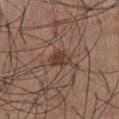Impression: This lesion was catalogued during total-body skin photography and was not selected for biopsy. Acquisition and patient details: The subject is a male approximately 55 years of age. On the abdomen. A region of skin cropped from a whole-body photographic capture, roughly 15 mm wide. Automated tile analysis of the lesion measured a footprint of about 4.5 mm², a shape eccentricity near 0.65, and a shape-asymmetry score of about 0.25 (0 = symmetric). And it measured a lesion-detection confidence of about 100/100. Measured at roughly 2.5 mm in maximum diameter. Imaged with white-light lighting.